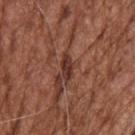{
  "biopsy_status": "not biopsied; imaged during a skin examination",
  "image": {
    "source": "total-body photography crop",
    "field_of_view_mm": 15
  },
  "lesion_size": {
    "long_diameter_mm_approx": 2.5
  },
  "site": "back",
  "lighting": "white-light",
  "patient": {
    "sex": "male",
    "age_approx": 75
  },
  "automated_metrics": {
    "area_mm2_approx": 2.5,
    "shape_asymmetry": 0.3,
    "border_irregularity_0_10": 3.0,
    "color_variation_0_10": 1.0,
    "peripheral_color_asymmetry": 0.5,
    "nevus_likeness_0_100": 0,
    "lesion_detection_confidence_0_100": 55
  }
}